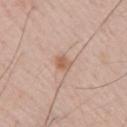– workup · no biopsy performed (imaged during a skin exam)
– automated lesion analysis · a lesion area of about 3.5 mm², an outline eccentricity of about 0.75 (0 = round, 1 = elongated), and a shape-asymmetry score of about 0.3 (0 = symmetric); a mean CIELAB color near L≈60 a*≈21 b*≈30, roughly 9 lightness units darker than nearby skin, and a lesion-to-skin contrast of about 7 (normalized; higher = more distinct); a lesion-detection confidence of about 100/100
– image source · 15 mm crop, total-body photography
– site · the arm
– patient · male, aged 48 to 52
– illumination · white-light
– diameter · about 2.5 mm